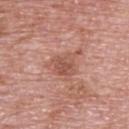{"biopsy_status": "not biopsied; imaged during a skin examination", "patient": {"sex": "male", "age_approx": 70}, "lesion_size": {"long_diameter_mm_approx": 4.5}, "site": "upper back", "image": {"source": "total-body photography crop", "field_of_view_mm": 15}}